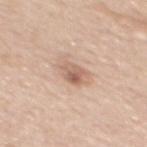Recorded during total-body skin imaging; not selected for excision or biopsy.
Imaged with white-light lighting.
Measured at roughly 3 mm in maximum diameter.
A male subject aged 48 to 52.
The lesion is on the mid back.
A 15 mm close-up extracted from a 3D total-body photography capture.